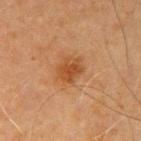Impression:
Part of a total-body skin-imaging series; this lesion was reviewed on a skin check and was not flagged for biopsy.
Context:
A region of skin cropped from a whole-body photographic capture, roughly 15 mm wide. The tile uses cross-polarized illumination. Located on the left upper arm. A male subject in their 70s.